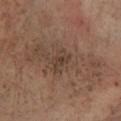Notes:
* notes: catalogued during a skin exam; not biopsied
* tile lighting: cross-polarized illumination
* patient: male, in their mid- to late 60s
* location: the left lower leg
* automated lesion analysis: a border-irregularity index near 4/10, a color-variation rating of about 3/10, and a peripheral color-asymmetry measure near 1
* image: ~15 mm tile from a whole-body skin photo